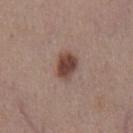Impression: This lesion was catalogued during total-body skin photography and was not selected for biopsy. Clinical summary: The lesion's longest dimension is about 3.5 mm. The total-body-photography lesion software estimated a border-irregularity index near 2/10, a color-variation rating of about 4/10, and radial color variation of about 1. It also reported an automated nevus-likeness rating near 95 out of 100 and a detector confidence of about 100 out of 100 that the crop contains a lesion. The lesion is on the chest. Imaged with white-light lighting. The subject is a male aged 53 to 57. A lesion tile, about 15 mm wide, cut from a 3D total-body photograph.